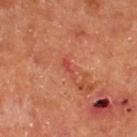Q: Is there a histopathology result?
A: total-body-photography surveillance lesion; no biopsy
Q: Automated lesion metrics?
A: an area of roughly 2 mm², an outline eccentricity of about 0.9 (0 = round, 1 = elongated), and a shape-asymmetry score of about 0.4 (0 = symmetric); a mean CIELAB color near L≈43 a*≈32 b*≈29 and a lesion–skin lightness drop of about 7; a border-irregularity index near 5/10 and radial color variation of about 0; a nevus-likeness score of about 0/100 and a lesion-detection confidence of about 95/100
Q: How large is the lesion?
A: ~2.5 mm (longest diameter)
Q: Illumination type?
A: cross-polarized illumination
Q: Where on the body is the lesion?
A: the upper back
Q: Who is the patient?
A: male, aged approximately 55
Q: What kind of image is this?
A: 15 mm crop, total-body photography